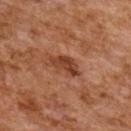follow-up = imaged on a skin check; not biopsied | image = ~15 mm crop, total-body skin-cancer survey | patient = female, about 60 years old | site = the upper back | illumination = cross-polarized illumination | TBP lesion metrics = an automated nevus-likeness rating near 25 out of 100 and a detector confidence of about 100 out of 100 that the crop contains a lesion | size = ~4 mm (longest diameter).